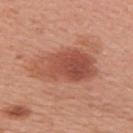- workup: catalogued during a skin exam; not biopsied